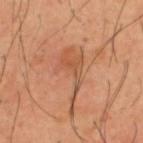biopsy_status: not biopsied; imaged during a skin examination
patient:
  sex: male
  age_approx: 40
image:
  source: total-body photography crop
  field_of_view_mm: 15
site: back